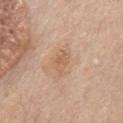No biopsy was performed on this lesion — it was imaged during a full skin examination and was not determined to be concerning.
Measured at roughly 2.5 mm in maximum diameter.
A close-up tile cropped from a whole-body skin photograph, about 15 mm across.
Imaged with white-light lighting.
An algorithmic analysis of the crop reported a color-variation rating of about 1.5/10 and a peripheral color-asymmetry measure near 0.5.
A male patient, in their mid- to late 70s.
The lesion is on the chest.